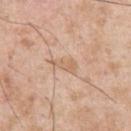Findings:
– workup · imaged on a skin check; not biopsied
– body site · the right upper arm
– diameter · ~3 mm (longest diameter)
– automated metrics · roughly 7 lightness units darker than nearby skin and a normalized border contrast of about 5; a border-irregularity rating of about 4/10 and internal color variation of about 1.5 on a 0–10 scale
– patient · male, aged approximately 55
– acquisition · ~15 mm crop, total-body skin-cancer survey
– tile lighting · white-light illumination